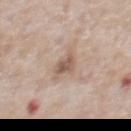Impression: This lesion was catalogued during total-body skin photography and was not selected for biopsy. Acquisition and patient details: A 15 mm close-up tile from a total-body photography series done for melanoma screening. A male subject, approximately 60 years of age. Automated image analysis of the tile measured an average lesion color of about L≈56 a*≈16 b*≈25 (CIELAB), a lesion–skin lightness drop of about 10, and a lesion-to-skin contrast of about 7 (normalized; higher = more distinct). And it measured a border-irregularity index near 3/10, a within-lesion color-variation index near 2.5/10, and radial color variation of about 1. On the front of the torso. Longest diameter approximately 3 mm.